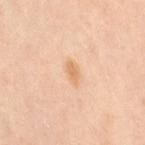  biopsy_status: not biopsied; imaged during a skin examination
  lighting: cross-polarized
  patient:
    sex: female
    age_approx: 40
  site: left leg
  image:
    source: total-body photography crop
    field_of_view_mm: 15